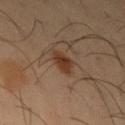Imaged during a routine full-body skin examination; the lesion was not biopsied and no histopathology is available. A male patient aged 38–42. On the arm. A 15 mm close-up tile from a total-body photography series done for melanoma screening. The lesion's longest dimension is about 3 mm.